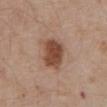This lesion was catalogued during total-body skin photography and was not selected for biopsy. A roughly 15 mm field-of-view crop from a total-body skin photograph. The lesion is located on the abdomen. The lesion's longest dimension is about 4 mm. The tile uses white-light illumination. The subject is a male roughly 80 years of age. An algorithmic analysis of the crop reported a lesion area of about 11 mm², an outline eccentricity of about 0.65 (0 = round, 1 = elongated), and a symmetry-axis asymmetry near 0.15. The analysis additionally found a mean CIELAB color near L≈47 a*≈21 b*≈29, a lesion–skin lightness drop of about 13, and a normalized lesion–skin contrast near 10. It also reported a border-irregularity index near 1.5/10 and internal color variation of about 3.5 on a 0–10 scale. The software also gave a nevus-likeness score of about 100/100 and lesion-presence confidence of about 100/100.